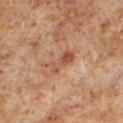Imaged during a routine full-body skin examination; the lesion was not biopsied and no histopathology is available. A 15 mm close-up tile from a total-body photography series done for melanoma screening. The lesion is on the leg. About 4 mm across. A male subject, approximately 60 years of age. Captured under cross-polarized illumination.